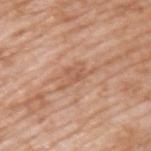<lesion>
  <biopsy_status>not biopsied; imaged during a skin examination</biopsy_status>
  <lighting>white-light</lighting>
  <patient>
    <sex>male</sex>
    <age_approx>60</age_approx>
  </patient>
  <site>back</site>
  <lesion_size>
    <long_diameter_mm_approx>4.5</long_diameter_mm_approx>
  </lesion_size>
  <automated_metrics>
    <area_mm2_approx>5.5</area_mm2_approx>
    <eccentricity>0.85</eccentricity>
    <shape_asymmetry>0.45</shape_asymmetry>
    <vs_skin_contrast_norm>5.5</vs_skin_contrast_norm>
    <peripheral_color_asymmetry>1.0</peripheral_color_asymmetry>
  </automated_metrics>
  <image>
    <source>total-body photography crop</source>
    <field_of_view_mm>15</field_of_view_mm>
  </image>
</lesion>